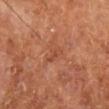  biopsy_status: not biopsied; imaged during a skin examination
  image:
    source: total-body photography crop
    field_of_view_mm: 15
  lesion_size:
    long_diameter_mm_approx: 3.0
  lighting: cross-polarized
  patient:
    sex: male
    age_approx: 70
  site: right lower leg